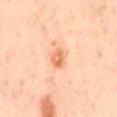The lesion was photographed on a routine skin check and not biopsied; there is no pathology result. A roughly 15 mm field-of-view crop from a total-body skin photograph. The subject is a male aged 63–67. An algorithmic analysis of the crop reported a footprint of about 4 mm² and a shape-asymmetry score of about 0.25 (0 = symmetric). The software also gave an average lesion color of about L≈71 a*≈27 b*≈41 (CIELAB), roughly 12 lightness units darker than nearby skin, and a lesion-to-skin contrast of about 7.5 (normalized; higher = more distinct). And it measured a border-irregularity index near 2.5/10 and radial color variation of about 1.5. And it measured an automated nevus-likeness rating near 85 out of 100 and lesion-presence confidence of about 100/100. The lesion is on the mid back.